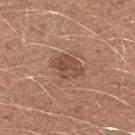biopsy status: imaged on a skin check; not biopsied
automated metrics: an area of roughly 7.5 mm², a shape eccentricity near 0.75, and a shape-asymmetry score of about 0.2 (0 = symmetric); a lesion–skin lightness drop of about 10; a nevus-likeness score of about 60/100 and a detector confidence of about 100 out of 100 that the crop contains a lesion
body site: the right upper arm
image: total-body-photography crop, ~15 mm field of view
size: ~4 mm (longest diameter)
subject: male, aged 23–27